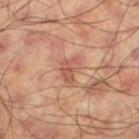Imaged during a routine full-body skin examination; the lesion was not biopsied and no histopathology is available.
Imaged with cross-polarized lighting.
The total-body-photography lesion software estimated a footprint of about 4.5 mm², a shape eccentricity near 0.8, and two-axis asymmetry of about 0.5. The software also gave a normalized lesion–skin contrast near 6.5. The analysis additionally found lesion-presence confidence of about 100/100.
Approximately 3 mm at its widest.
A roughly 15 mm field-of-view crop from a total-body skin photograph.
A male patient in their 60s.
The lesion is on the left thigh.